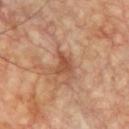The lesion was photographed on a routine skin check and not biopsied; there is no pathology result. The tile uses cross-polarized illumination. Longest diameter approximately 3 mm. A close-up tile cropped from a whole-body skin photograph, about 15 mm across. The patient is a male aged 58–62. The lesion is located on the chest.